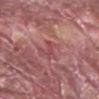Case summary:
* subject · male, in their 40s
* body site · the arm
* size · ~2.5 mm (longest diameter)
* acquisition · 15 mm crop, total-body photography
* tile lighting · white-light illumination